Cropped from a total-body skin-imaging series; the visible field is about 15 mm. The lesion is located on the left lower leg. A female patient approximately 45 years of age — 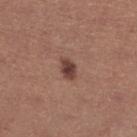The lesion was biopsied, and histopathology showed a junctional melanocytic nevus.